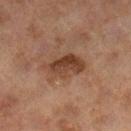biopsy status = imaged on a skin check; not biopsied
image = ~15 mm crop, total-body skin-cancer survey
lighting = cross-polarized illumination
body site = the right lower leg
lesion size = ~4.5 mm (longest diameter)
patient = female, approximately 60 years of age